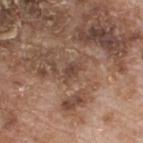The lesion was tiled from a total-body skin photograph and was not biopsied.
A male subject, approximately 80 years of age.
Automated tile analysis of the lesion measured a footprint of about 3 mm², an outline eccentricity of about 0.85 (0 = round, 1 = elongated), and a symmetry-axis asymmetry near 0.3. The software also gave a mean CIELAB color near L≈44 a*≈17 b*≈26. The software also gave a border-irregularity index near 3.5/10.
Captured under white-light illumination.
The recorded lesion diameter is about 3 mm.
From the back.
This image is a 15 mm lesion crop taken from a total-body photograph.